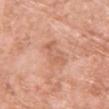This lesion was catalogued during total-body skin photography and was not selected for biopsy.
An algorithmic analysis of the crop reported a mean CIELAB color near L≈62 a*≈24 b*≈32 and a normalized border contrast of about 5. It also reported a border-irregularity index near 4.5/10 and a peripheral color-asymmetry measure near 1.
Located on the front of the torso.
A region of skin cropped from a whole-body photographic capture, roughly 15 mm wide.
The recorded lesion diameter is about 4.5 mm.
A female subject, about 70 years old.